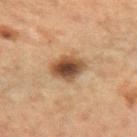A close-up tile cropped from a whole-body skin photograph, about 15 mm across. Measured at roughly 4 mm in maximum diameter. A female patient aged 38 to 42. Located on the left forearm.